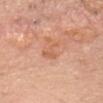workup = no biopsy performed (imaged during a skin exam)
diameter = ≈3 mm
body site = the head or neck
acquisition = ~15 mm crop, total-body skin-cancer survey
subject = male, about 40 years old